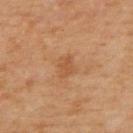Imaged during a routine full-body skin examination; the lesion was not biopsied and no histopathology is available.
The lesion is on the upper back.
A roughly 15 mm field-of-view crop from a total-body skin photograph.
A female patient, aged approximately 70.